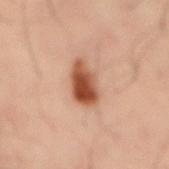The lesion was photographed on a routine skin check and not biopsied; there is no pathology result. A male patient roughly 50 years of age. The lesion's longest dimension is about 4.5 mm. Cropped from a whole-body photographic skin survey; the tile spans about 15 mm. The lesion-visualizer software estimated a border-irregularity index near 2.5/10, internal color variation of about 8 on a 0–10 scale, and a peripheral color-asymmetry measure near 3. The analysis additionally found a nevus-likeness score of about 100/100 and a detector confidence of about 100 out of 100 that the crop contains a lesion. On the mid back.